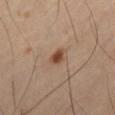Clinical impression: No biopsy was performed on this lesion — it was imaged during a full skin examination and was not determined to be concerning. Image and clinical context: A male subject, aged approximately 60. From the abdomen. Longest diameter approximately 2 mm. A lesion tile, about 15 mm wide, cut from a 3D total-body photograph. An algorithmic analysis of the crop reported a footprint of about 3 mm² and a shape-asymmetry score of about 0.35 (0 = symmetric). The software also gave an average lesion color of about L≈43 a*≈20 b*≈30 (CIELAB), about 12 CIELAB-L* units darker than the surrounding skin, and a normalized lesion–skin contrast near 10. It also reported a border-irregularity rating of about 2.5/10, internal color variation of about 2 on a 0–10 scale, and peripheral color asymmetry of about 0.5. The analysis additionally found an automated nevus-likeness rating near 95 out of 100.